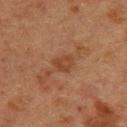Q: Who is the patient?
A: female, roughly 55 years of age
Q: What lighting was used for the tile?
A: cross-polarized illumination
Q: What is the imaging modality?
A: total-body-photography crop, ~15 mm field of view
Q: How large is the lesion?
A: about 2.5 mm
Q: Lesion location?
A: the back